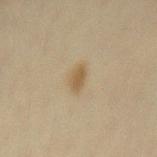<lesion>
  <biopsy_status>not biopsied; imaged during a skin examination</biopsy_status>
  <automated_metrics>
    <area_mm2_approx>4.0</area_mm2_approx>
    <eccentricity>0.75</eccentricity>
    <shape_asymmetry>0.15</shape_asymmetry>
    <nevus_likeness_0_100>90</nevus_likeness_0_100>
    <lesion_detection_confidence_0_100>100</lesion_detection_confidence_0_100>
  </automated_metrics>
  <image>
    <source>total-body photography crop</source>
    <field_of_view_mm>15</field_of_view_mm>
  </image>
  <patient>
    <sex>female</sex>
    <age_approx>45</age_approx>
  </patient>
  <site>mid back</site>
  <lesion_size>
    <long_diameter_mm_approx>2.5</long_diameter_mm_approx>
  </lesion_size>
</lesion>